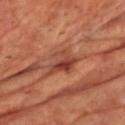Q: Is there a histopathology result?
A: catalogued during a skin exam; not biopsied
Q: What is the imaging modality?
A: ~15 mm crop, total-body skin-cancer survey
Q: Where on the body is the lesion?
A: the chest
Q: Patient demographics?
A: male, in their mid-60s